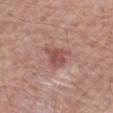{
  "biopsy_status": "not biopsied; imaged during a skin examination",
  "automated_metrics": {
    "area_mm2_approx": 7.5,
    "eccentricity": 0.55,
    "cielab_L": 52,
    "cielab_a": 22,
    "cielab_b": 25,
    "vs_skin_darker_L": 10.0
  },
  "site": "right forearm",
  "lighting": "white-light",
  "lesion_size": {
    "long_diameter_mm_approx": 4.0
  },
  "patient": {
    "sex": "male",
    "age_approx": 60
  },
  "image": {
    "source": "total-body photography crop",
    "field_of_view_mm": 15
  }
}